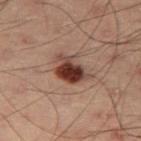Q: Is there a histopathology result?
A: total-body-photography surveillance lesion; no biopsy
Q: What are the patient's age and sex?
A: male, roughly 55 years of age
Q: What is the anatomic site?
A: the right thigh
Q: What is the imaging modality?
A: ~15 mm crop, total-body skin-cancer survey
Q: Automated lesion metrics?
A: an average lesion color of about L≈30 a*≈18 b*≈21 (CIELAB), a lesion–skin lightness drop of about 14, and a lesion-to-skin contrast of about 12.5 (normalized; higher = more distinct); internal color variation of about 7.5 on a 0–10 scale; a classifier nevus-likeness of about 100/100 and a detector confidence of about 100 out of 100 that the crop contains a lesion
Q: What lighting was used for the tile?
A: cross-polarized
Q: Lesion size?
A: ≈4 mm